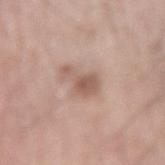The lesion was tiled from a total-body skin photograph and was not biopsied. The lesion's longest dimension is about 4 mm. The lesion is on the left upper arm. A roughly 15 mm field-of-view crop from a total-body skin photograph. The subject is a male in their 70s. This is a white-light tile.